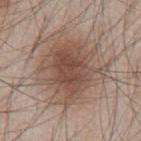Q: Was a biopsy performed?
A: no biopsy performed (imaged during a skin exam)